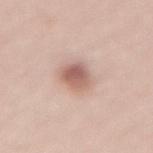<record>
<biopsy_status>not biopsied; imaged during a skin examination</biopsy_status>
<image>
  <source>total-body photography crop</source>
  <field_of_view_mm>15</field_of_view_mm>
</image>
<patient>
  <sex>male</sex>
  <age_approx>55</age_approx>
</patient>
<site>lower back</site>
</record>